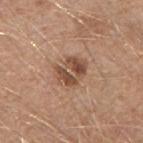tile lighting — white-light illumination
patient — male, roughly 40 years of age
site — the right upper arm
imaging modality — total-body-photography crop, ~15 mm field of view